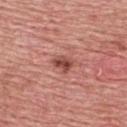notes: total-body-photography surveillance lesion; no biopsy | lesion size: about 2.5 mm | imaging modality: ~15 mm tile from a whole-body skin photo | body site: the upper back | subject: male, approximately 60 years of age | automated lesion analysis: a footprint of about 4 mm², a shape eccentricity near 0.6, and two-axis asymmetry of about 0.3; an average lesion color of about L≈48 a*≈27 b*≈27 (CIELAB) and a lesion-to-skin contrast of about 8.5 (normalized; higher = more distinct); lesion-presence confidence of about 100/100.